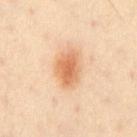Captured during whole-body skin photography for melanoma surveillance; the lesion was not biopsied.
The recorded lesion diameter is about 4.5 mm.
A 15 mm close-up tile from a total-body photography series done for melanoma screening.
From the chest.
A male subject roughly 45 years of age.